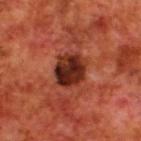Impression: Recorded during total-body skin imaging; not selected for excision or biopsy. Clinical summary: The tile uses cross-polarized illumination. On the upper back. The patient is a male approximately 70 years of age. Measured at roughly 4 mm in maximum diameter. A 15 mm crop from a total-body photograph taken for skin-cancer surveillance.